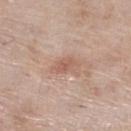Imaged during a routine full-body skin examination; the lesion was not biopsied and no histopathology is available. The lesion's longest dimension is about 3.5 mm. The lesion-visualizer software estimated a footprint of about 5 mm² and a shape-asymmetry score of about 0.35 (0 = symmetric). The analysis additionally found a lesion color around L≈59 a*≈20 b*≈27 in CIELAB, a lesion–skin lightness drop of about 8, and a normalized border contrast of about 5.5. The software also gave a detector confidence of about 100 out of 100 that the crop contains a lesion. A roughly 15 mm field-of-view crop from a total-body skin photograph. The subject is a female aged 73 to 77. Located on the right lower leg. Captured under white-light illumination.